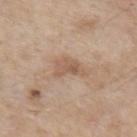tile lighting: white-light illumination | image: ~15 mm tile from a whole-body skin photo | patient: male, in their 60s | automated lesion analysis: a lesion area of about 5.5 mm², a shape eccentricity near 0.85, and two-axis asymmetry of about 0.45; a nevus-likeness score of about 0/100 | lesion size: ~4 mm (longest diameter) | body site: the left upper arm.